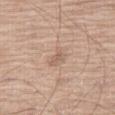The lesion is located on the right thigh.
The patient is a male roughly 70 years of age.
A lesion tile, about 15 mm wide, cut from a 3D total-body photograph.